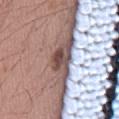notes: imaged on a skin check; not biopsied
patient: male, aged 33–37
automated lesion analysis: an area of roughly 4.5 mm², a shape eccentricity near 0.7, and a shape-asymmetry score of about 0.35 (0 = symmetric); a lesion color around L≈46 a*≈18 b*≈20 in CIELAB and about 21 CIELAB-L* units darker than the surrounding skin; border irregularity of about 4 on a 0–10 scale
acquisition: 15 mm crop, total-body photography
lesion size: ≈3 mm
illumination: white-light
location: the mid back